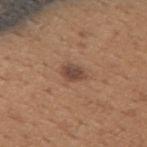workup=catalogued during a skin exam; not biopsied | illumination=white-light | lesion size=≈3 mm | image-analysis metrics=a lesion area of about 4 mm², a shape eccentricity near 0.75, and two-axis asymmetry of about 0.3; an average lesion color of about L≈45 a*≈19 b*≈26 (CIELAB) and a lesion–skin lightness drop of about 11; an automated nevus-likeness rating near 75 out of 100 | image source=~15 mm crop, total-body skin-cancer survey | patient=male, in their mid-60s | body site=the back.